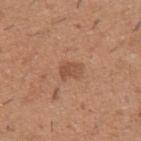| key | value |
|---|---|
| follow-up | no biopsy performed (imaged during a skin exam) |
| subject | male, roughly 40 years of age |
| image source | ~15 mm crop, total-body skin-cancer survey |
| lighting | white-light |
| diameter | ~2.5 mm (longest diameter) |
| site | the left upper arm |